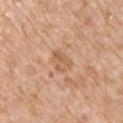Assessment: Recorded during total-body skin imaging; not selected for excision or biopsy. Image and clinical context: This is a white-light tile. The recorded lesion diameter is about 3 mm. A 15 mm crop from a total-body photograph taken for skin-cancer surveillance. A male subject, roughly 65 years of age. The lesion is on the chest.